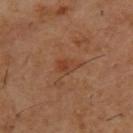Part of a total-body skin-imaging series; this lesion was reviewed on a skin check and was not flagged for biopsy. A 15 mm close-up tile from a total-body photography series done for melanoma screening. This is a cross-polarized tile. From the upper back. A male subject, aged around 55.